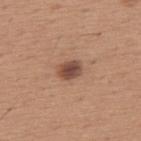Q: Is there a histopathology result?
A: total-body-photography surveillance lesion; no biopsy
Q: What is the imaging modality?
A: total-body-photography crop, ~15 mm field of view
Q: What are the patient's age and sex?
A: male, approximately 65 years of age
Q: Lesion size?
A: about 2.5 mm
Q: Lesion location?
A: the upper back
Q: How was the tile lit?
A: white-light illumination